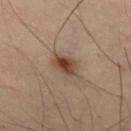Impression:
No biopsy was performed on this lesion — it was imaged during a full skin examination and was not determined to be concerning.
Context:
About 3 mm across. A male patient, aged 28–32. The lesion is on the chest. A close-up tile cropped from a whole-body skin photograph, about 15 mm across. Automated image analysis of the tile measured a lesion–skin lightness drop of about 10.